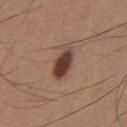workup: total-body-photography surveillance lesion; no biopsy
site: the chest
illumination: white-light
acquisition: ~15 mm crop, total-body skin-cancer survey
size: about 4 mm
subject: male, aged approximately 35